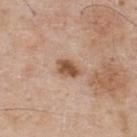{"biopsy_status": "not biopsied; imaged during a skin examination", "patient": {"sex": "male", "age_approx": 60}, "image": {"source": "total-body photography crop", "field_of_view_mm": 15}, "site": "upper back", "automated_metrics": {"cielab_L": 53, "cielab_a": 20, "cielab_b": 31, "vs_skin_darker_L": 14.0, "nevus_likeness_0_100": 90, "lesion_detection_confidence_0_100": 100}, "lighting": "white-light"}